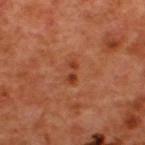follow-up: catalogued during a skin exam; not biopsied | patient: male, aged 48 to 52 | image: ~15 mm tile from a whole-body skin photo | lighting: cross-polarized | lesion size: about 2.5 mm | anatomic site: the back.